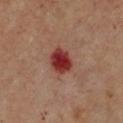| feature | finding |
|---|---|
| biopsy status | imaged on a skin check; not biopsied |
| site | the chest |
| imaging modality | ~15 mm crop, total-body skin-cancer survey |
| subject | female, about 45 years old |